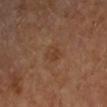Notes:
- workup: catalogued during a skin exam; not biopsied
- site: the arm
- patient: roughly 60 years of age
- lighting: cross-polarized
- imaging modality: total-body-photography crop, ~15 mm field of view